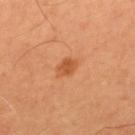Clinical impression: This lesion was catalogued during total-body skin photography and was not selected for biopsy. Acquisition and patient details: Cropped from a total-body skin-imaging series; the visible field is about 15 mm. A male subject, aged approximately 65. Located on the mid back. Imaged with cross-polarized lighting.